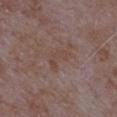The lesion was tiled from a total-body skin photograph and was not biopsied. Captured under white-light illumination. This image is a 15 mm lesion crop taken from a total-body photograph. On the left upper arm. A male patient aged 63–67. The recorded lesion diameter is about 3 mm.